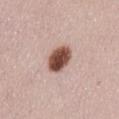Captured during whole-body skin photography for melanoma surveillance; the lesion was not biopsied. A female patient in their 30s. From the abdomen. Imaged with white-light lighting. This image is a 15 mm lesion crop taken from a total-body photograph. An algorithmic analysis of the crop reported about 22 CIELAB-L* units darker than the surrounding skin and a normalized border contrast of about 14.5. It also reported a nevus-likeness score of about 100/100.